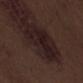No biopsy was performed on this lesion — it was imaged during a full skin examination and was not determined to be concerning. Imaged with white-light lighting. A region of skin cropped from a whole-body photographic capture, roughly 15 mm wide. Located on the left thigh. The subject is a male aged around 70.